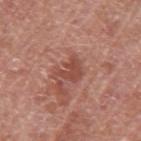Impression:
Part of a total-body skin-imaging series; this lesion was reviewed on a skin check and was not flagged for biopsy.